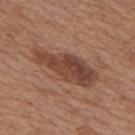notes: no biopsy performed (imaged during a skin exam)
location: the back
image source: ~15 mm crop, total-body skin-cancer survey
size: about 8 mm
patient: male, approximately 65 years of age